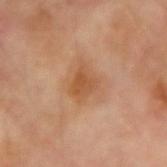{
  "site": "right forearm",
  "image": {
    "source": "total-body photography crop",
    "field_of_view_mm": 15
  },
  "patient": {
    "sex": "male",
    "age_approx": 70
  }
}